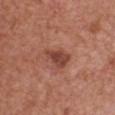imaging modality: ~15 mm crop, total-body skin-cancer survey | TBP lesion metrics: a lesion area of about 6 mm², an outline eccentricity of about 0.8 (0 = round, 1 = elongated), and a shape-asymmetry score of about 0.4 (0 = symmetric); a lesion color around L≈44 a*≈25 b*≈28 in CIELAB and a normalized border contrast of about 8.5; border irregularity of about 4 on a 0–10 scale, internal color variation of about 3 on a 0–10 scale, and peripheral color asymmetry of about 1; an automated nevus-likeness rating near 45 out of 100 and a lesion-detection confidence of about 100/100 | lesion diameter: ≈4 mm | lighting: white-light illumination | subject: male, about 65 years old | anatomic site: the front of the torso.